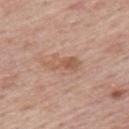Q: Is there a histopathology result?
A: catalogued during a skin exam; not biopsied
Q: Who is the patient?
A: male, aged around 75
Q: What did automated image analysis measure?
A: border irregularity of about 3 on a 0–10 scale, internal color variation of about 5 on a 0–10 scale, and peripheral color asymmetry of about 2
Q: Where on the body is the lesion?
A: the mid back
Q: Illumination type?
A: white-light
Q: Lesion size?
A: ≈4 mm
Q: What kind of image is this?
A: ~15 mm tile from a whole-body skin photo